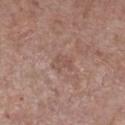Captured during whole-body skin photography for melanoma surveillance; the lesion was not biopsied.
The subject is a male about 55 years old.
The tile uses white-light illumination.
The lesion is located on the right lower leg.
A 15 mm close-up tile from a total-body photography series done for melanoma screening.